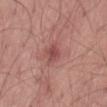Impression: No biopsy was performed on this lesion — it was imaged during a full skin examination and was not determined to be concerning. Context: This is a white-light tile. About 2.5 mm across. A 15 mm close-up extracted from a 3D total-body photography capture. A male subject, aged approximately 55. Located on the leg.